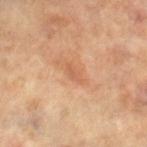From the left leg.
The recorded lesion diameter is about 2.5 mm.
A close-up tile cropped from a whole-body skin photograph, about 15 mm across.
The total-body-photography lesion software estimated a lesion area of about 2.5 mm², an eccentricity of roughly 0.9, and two-axis asymmetry of about 0.25. The software also gave an average lesion color of about L≈58 a*≈24 b*≈35 (CIELAB), roughly 7 lightness units darker than nearby skin, and a normalized border contrast of about 5.
The patient is a female aged approximately 65.